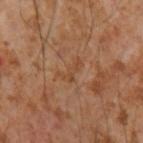Impression: No biopsy was performed on this lesion — it was imaged during a full skin examination and was not determined to be concerning. Context: From the right forearm. A region of skin cropped from a whole-body photographic capture, roughly 15 mm wide. The tile uses cross-polarized illumination. The lesion-visualizer software estimated a footprint of about 4 mm², an outline eccentricity of about 0.85 (0 = round, 1 = elongated), and a shape-asymmetry score of about 0.6 (0 = symmetric). The software also gave a border-irregularity index near 7/10, a within-lesion color-variation index near 0/10, and peripheral color asymmetry of about 0. The software also gave a nevus-likeness score of about 0/100 and a detector confidence of about 100 out of 100 that the crop contains a lesion. The patient is a male aged approximately 55.